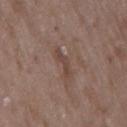A region of skin cropped from a whole-body photographic capture, roughly 15 mm wide.
A male patient about 50 years old.
The lesion is on the right upper arm.
The lesion-visualizer software estimated an outline eccentricity of about 0.9 (0 = round, 1 = elongated) and a symmetry-axis asymmetry near 0.55. And it measured a mean CIELAB color near L≈43 a*≈17 b*≈23, about 7 CIELAB-L* units darker than the surrounding skin, and a normalized border contrast of about 6. The software also gave a nevus-likeness score of about 0/100.